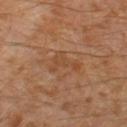Clinical impression:
Captured during whole-body skin photography for melanoma surveillance; the lesion was not biopsied.